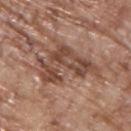Imaged during a routine full-body skin examination; the lesion was not biopsied and no histopathology is available. A 15 mm close-up tile from a total-body photography series done for melanoma screening. On the back. A male subject, approximately 70 years of age.